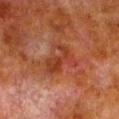The lesion was tiled from a total-body skin photograph and was not biopsied.
The total-body-photography lesion software estimated a footprint of about 9.5 mm² and two-axis asymmetry of about 0.45. The software also gave a mean CIELAB color near L≈31 a*≈25 b*≈28, roughly 7 lightness units darker than nearby skin, and a lesion-to-skin contrast of about 6.5 (normalized; higher = more distinct). And it measured a border-irregularity index near 7/10, a within-lesion color-variation index near 3/10, and peripheral color asymmetry of about 1.
A close-up tile cropped from a whole-body skin photograph, about 15 mm across.
The lesion is located on the left lower leg.
A male patient, aged around 80.
The lesion's longest dimension is about 4.5 mm.
This is a cross-polarized tile.